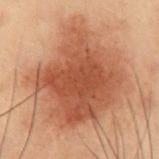Clinical impression: This lesion was catalogued during total-body skin photography and was not selected for biopsy. Image and clinical context: Located on the abdomen. A male subject aged 53–57. Longest diameter approximately 10 mm. Cropped from a whole-body photographic skin survey; the tile spans about 15 mm.